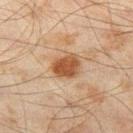<lesion>
  <biopsy_status>not biopsied; imaged during a skin examination</biopsy_status>
  <automated_metrics>
    <eccentricity>0.6</eccentricity>
    <shape_asymmetry>0.2</shape_asymmetry>
    <border_irregularity_0_10>2.0</border_irregularity_0_10>
    <peripheral_color_asymmetry>1.0</peripheral_color_asymmetry>
  </automated_metrics>
  <lesion_size>
    <long_diameter_mm_approx>3.5</long_diameter_mm_approx>
  </lesion_size>
  <patient>
    <sex>male</sex>
    <age_approx>45</age_approx>
  </patient>
  <site>left thigh</site>
  <image>
    <source>total-body photography crop</source>
    <field_of_view_mm>15</field_of_view_mm>
  </image>
</lesion>